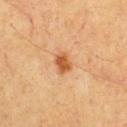| key | value |
|---|---|
| biopsy status | imaged on a skin check; not biopsied |
| image-analysis metrics | a mean CIELAB color near L≈50 a*≈24 b*≈38 and a lesion–skin lightness drop of about 11; an automated nevus-likeness rating near 95 out of 100 and a detector confidence of about 100 out of 100 that the crop contains a lesion |
| image source | ~15 mm crop, total-body skin-cancer survey |
| location | the chest |
| diameter | ≈2.5 mm |
| patient | male, in their mid- to late 50s |